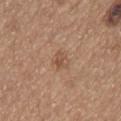Captured during whole-body skin photography for melanoma surveillance; the lesion was not biopsied.
Approximately 2.5 mm at its widest.
Cropped from a whole-body photographic skin survey; the tile spans about 15 mm.
Imaged with white-light lighting.
A male patient about 65 years old.
The lesion is on the abdomen.